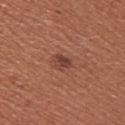notes: total-body-photography surveillance lesion; no biopsy
tile lighting: white-light illumination
size: ~2.5 mm (longest diameter)
subject: female, aged around 35
anatomic site: the left upper arm
image: ~15 mm tile from a whole-body skin photo